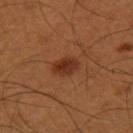notes: total-body-photography surveillance lesion; no biopsy
image-analysis metrics: a footprint of about 5 mm², an eccentricity of roughly 0.75, and two-axis asymmetry of about 0.25; a mean CIELAB color near L≈32 a*≈25 b*≈32, about 10 CIELAB-L* units darker than the surrounding skin, and a normalized border contrast of about 8.5; a detector confidence of about 100 out of 100 that the crop contains a lesion
patient: male, aged 48 to 52
lighting: cross-polarized illumination
lesion diameter: about 3 mm
site: the right thigh
acquisition: ~15 mm tile from a whole-body skin photo